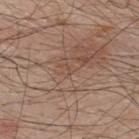Assessment: This lesion was catalogued during total-body skin photography and was not selected for biopsy. Acquisition and patient details: The tile uses white-light illumination. A 15 mm crop from a total-body photograph taken for skin-cancer surveillance. Automated image analysis of the tile measured a lesion–skin lightness drop of about 5 and a lesion-to-skin contrast of about 4 (normalized; higher = more distinct). And it measured border irregularity of about 4 on a 0–10 scale, a within-lesion color-variation index near 0/10, and a peripheral color-asymmetry measure near 0. A male subject, about 50 years old. About 1 mm across. On the upper back.